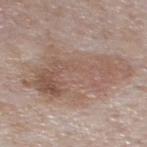The lesion was tiled from a total-body skin photograph and was not biopsied.
A close-up tile cropped from a whole-body skin photograph, about 15 mm across.
The total-body-photography lesion software estimated an eccentricity of roughly 0.8 and a shape-asymmetry score of about 0.3 (0 = symmetric). The software also gave lesion-presence confidence of about 100/100.
From the mid back.
The recorded lesion diameter is about 10.5 mm.
The patient is a male aged around 75.